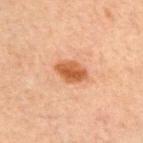<case>
<biopsy_status>not biopsied; imaged during a skin examination</biopsy_status>
<patient>
  <sex>male</sex>
  <age_approx>60</age_approx>
</patient>
<lesion_size>
  <long_diameter_mm_approx>4.0</long_diameter_mm_approx>
</lesion_size>
<lighting>cross-polarized</lighting>
<image>
  <source>total-body photography crop</source>
  <field_of_view_mm>15</field_of_view_mm>
</image>
<site>chest</site>
</case>